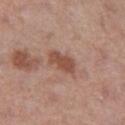Part of a total-body skin-imaging series; this lesion was reviewed on a skin check and was not flagged for biopsy. Cropped from a total-body skin-imaging series; the visible field is about 15 mm. Automated tile analysis of the lesion measured roughly 10 lightness units darker than nearby skin and a normalized border contrast of about 8. The software also gave an automated nevus-likeness rating near 65 out of 100 and lesion-presence confidence of about 100/100. Located on the left thigh. The subject is a male approximately 70 years of age.